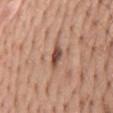The lesion was photographed on a routine skin check and not biopsied; there is no pathology result. A region of skin cropped from a whole-body photographic capture, roughly 15 mm wide. The tile uses white-light illumination. Longest diameter approximately 3.5 mm. The lesion is located on the mid back. The patient is a male aged approximately 60.